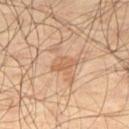Assessment: Captured during whole-body skin photography for melanoma surveillance; the lesion was not biopsied. Image and clinical context: The recorded lesion diameter is about 3.5 mm. The tile uses cross-polarized illumination. Located on the right thigh. The patient is a male aged 53–57. A roughly 15 mm field-of-view crop from a total-body skin photograph.